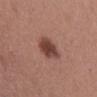• biopsy status: imaged on a skin check; not biopsied
• patient: female, aged 33 to 37
• automated metrics: an outline eccentricity of about 0.75 (0 = round, 1 = elongated) and a symmetry-axis asymmetry near 0.2; a mean CIELAB color near L≈42 a*≈22 b*≈24, roughly 14 lightness units darker than nearby skin, and a normalized border contrast of about 10.5; a border-irregularity rating of about 2/10, a within-lesion color-variation index near 3/10, and a peripheral color-asymmetry measure near 0.5; a nevus-likeness score of about 95/100 and a detector confidence of about 100 out of 100 that the crop contains a lesion
• imaging modality: total-body-photography crop, ~15 mm field of view
• anatomic site: the abdomen